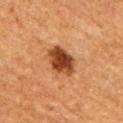Recorded during total-body skin imaging; not selected for excision or biopsy. Automated tile analysis of the lesion measured an area of roughly 9.5 mm², a shape eccentricity near 0.6, and a symmetry-axis asymmetry near 0.2. And it measured an average lesion color of about L≈42 a*≈27 b*≈37 (CIELAB), a lesion–skin lightness drop of about 17, and a normalized lesion–skin contrast near 12. And it measured a border-irregularity index near 2/10, internal color variation of about 6 on a 0–10 scale, and a peripheral color-asymmetry measure near 1.5. The analysis additionally found a detector confidence of about 100 out of 100 that the crop contains a lesion. The tile uses cross-polarized illumination. On the right upper arm. A roughly 15 mm field-of-view crop from a total-body skin photograph. The patient is a female roughly 55 years of age.